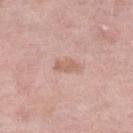Captured during whole-body skin photography for melanoma surveillance; the lesion was not biopsied. Located on the left lower leg. Cropped from a whole-body photographic skin survey; the tile spans about 15 mm. Imaged with white-light lighting. The patient is a female approximately 70 years of age. Measured at roughly 3 mm in maximum diameter.